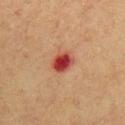Findings:
* notes — catalogued during a skin exam; not biopsied
* image source — 15 mm crop, total-body photography
* subject — male, aged around 60
* lighting — cross-polarized
* diameter — about 3 mm